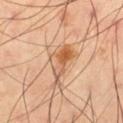The lesion was tiled from a total-body skin photograph and was not biopsied. A region of skin cropped from a whole-body photographic capture, roughly 15 mm wide. A male subject roughly 65 years of age. Located on the left thigh.